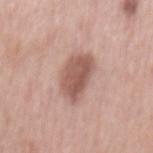  biopsy_status: not biopsied; imaged during a skin examination
  patient:
    sex: male
    age_approx: 65
  lighting: white-light
  lesion_size:
    long_diameter_mm_approx: 5.0
  site: mid back
  image:
    source: total-body photography crop
    field_of_view_mm: 15
  automated_metrics:
    area_mm2_approx: 13.0
    eccentricity: 0.8
    nevus_likeness_0_100: 60
    lesion_detection_confidence_0_100: 100